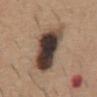<lesion>
  <biopsy_status>not biopsied; imaged during a skin examination</biopsy_status>
  <site>abdomen</site>
  <patient>
    <sex>male</sex>
    <age_approx>60</age_approx>
  </patient>
  <image>
    <source>total-body photography crop</source>
    <field_of_view_mm>15</field_of_view_mm>
  </image>
</lesion>